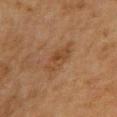Assessment:
No biopsy was performed on this lesion — it was imaged during a full skin examination and was not determined to be concerning.
Clinical summary:
A lesion tile, about 15 mm wide, cut from a 3D total-body photograph. Located on the right upper arm. The patient is a female aged 53 to 57.